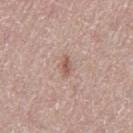Q: Was a biopsy performed?
A: imaged on a skin check; not biopsied
Q: How was the tile lit?
A: white-light illumination
Q: What kind of image is this?
A: ~15 mm tile from a whole-body skin photo
Q: Who is the patient?
A: female, in their mid- to late 60s
Q: Lesion location?
A: the left thigh